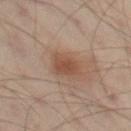No biopsy was performed on this lesion — it was imaged during a full skin examination and was not determined to be concerning.
The lesion is on the leg.
Cropped from a total-body skin-imaging series; the visible field is about 15 mm.
A male subject, aged around 50.